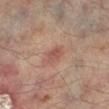The lesion is on the right lower leg.
A 15 mm close-up tile from a total-body photography series done for melanoma screening.
Captured under cross-polarized illumination.
A male patient aged around 65.